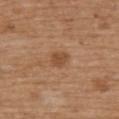{
  "biopsy_status": "not biopsied; imaged during a skin examination",
  "image": {
    "source": "total-body photography crop",
    "field_of_view_mm": 15
  },
  "lighting": "white-light",
  "patient": {
    "sex": "male",
    "age_approx": 70
  },
  "lesion_size": {
    "long_diameter_mm_approx": 2.5
  },
  "site": "upper back"
}